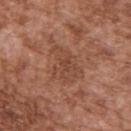Captured during whole-body skin photography for melanoma surveillance; the lesion was not biopsied. Automated tile analysis of the lesion measured an area of roughly 12 mm² and a symmetry-axis asymmetry near 0.35. And it measured a lesion color around L≈46 a*≈23 b*≈30 in CIELAB, roughly 7 lightness units darker than nearby skin, and a lesion-to-skin contrast of about 5 (normalized; higher = more distinct). The analysis additionally found an automated nevus-likeness rating near 0 out of 100. This is a white-light tile. On the upper back. The subject is a male approximately 75 years of age. Approximately 5 mm at its widest. A lesion tile, about 15 mm wide, cut from a 3D total-body photograph.